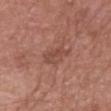Notes:
– workup · catalogued during a skin exam; not biopsied
– lighting · white-light
– patient · male, aged 63 to 67
– lesion size · about 3.5 mm
– imaging modality · ~15 mm tile from a whole-body skin photo
– body site · the head or neck
– automated lesion analysis · an average lesion color of about L≈47 a*≈23 b*≈27 (CIELAB) and a lesion–skin lightness drop of about 7; a border-irregularity rating of about 4/10 and a peripheral color-asymmetry measure near 1